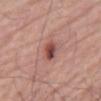follow-up: catalogued during a skin exam; not biopsied
image source: ~15 mm crop, total-body skin-cancer survey
body site: the abdomen
size: ≈3 mm
tile lighting: white-light illumination
patient: male, aged 78 to 82